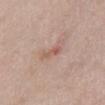Impression:
Imaged during a routine full-body skin examination; the lesion was not biopsied and no histopathology is available.
Acquisition and patient details:
On the abdomen. Approximately 3 mm at its widest. A 15 mm close-up tile from a total-body photography series done for melanoma screening. This is a white-light tile. The subject is a male about 65 years old.